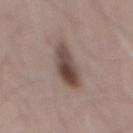The total-body-photography lesion software estimated a lesion area of about 11 mm² and a symmetry-axis asymmetry near 0.25. And it measured internal color variation of about 7 on a 0–10 scale and peripheral color asymmetry of about 2.5.
Imaged with white-light lighting.
This image is a 15 mm lesion crop taken from a total-body photograph.
The lesion's longest dimension is about 4.5 mm.
The lesion is located on the mid back.
A male patient, aged approximately 70.